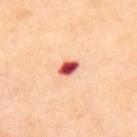Case summary:
* workup — imaged on a skin check; not biopsied
* anatomic site — the upper back
* image — total-body-photography crop, ~15 mm field of view
* patient — female, roughly 55 years of age
* illumination — cross-polarized illumination
* TBP lesion metrics — a lesion color around L≈59 a*≈39 b*≈33 in CIELAB and a normalized lesion–skin contrast near 14